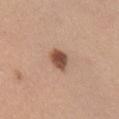{
  "biopsy_status": "not biopsied; imaged during a skin examination",
  "image": {
    "source": "total-body photography crop",
    "field_of_view_mm": 15
  },
  "patient": {
    "sex": "female",
    "age_approx": 30
  },
  "automated_metrics": {
    "area_mm2_approx": 5.0,
    "eccentricity": 0.65,
    "shape_asymmetry": 0.2
  },
  "site": "right upper arm"
}